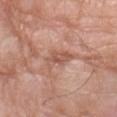This lesion was catalogued during total-body skin photography and was not selected for biopsy.
From the arm.
The lesion-visualizer software estimated border irregularity of about 4 on a 0–10 scale and peripheral color asymmetry of about 1.
A male patient, in their 60s.
A close-up tile cropped from a whole-body skin photograph, about 15 mm across.
Approximately 4 mm at its widest.